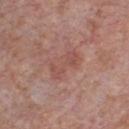No biopsy was performed on this lesion — it was imaged during a full skin examination and was not determined to be concerning. The lesion is on the chest. This is a white-light tile. Automated image analysis of the tile measured a lesion area of about 5.5 mm², an outline eccentricity of about 0.9 (0 = round, 1 = elongated), and two-axis asymmetry of about 0.35. And it measured an average lesion color of about L≈50 a*≈23 b*≈25 (CIELAB), roughly 6 lightness units darker than nearby skin, and a lesion-to-skin contrast of about 5 (normalized; higher = more distinct). And it measured a border-irregularity rating of about 5.5/10 and a within-lesion color-variation index near 2.5/10. And it measured a nevus-likeness score of about 0/100 and a lesion-detection confidence of about 100/100. Cropped from a total-body skin-imaging series; the visible field is about 15 mm. A male subject approximately 60 years of age.